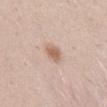biopsy status = no biopsy performed (imaged during a skin exam)
acquisition = 15 mm crop, total-body photography
diameter = about 2.5 mm
illumination = white-light
site = the abdomen
subject = male, aged 33–37